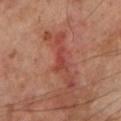The patient is a male approximately 70 years of age. The recorded lesion diameter is about 3 mm. This is a cross-polarized tile. The lesion is on the left lower leg. Cropped from a total-body skin-imaging series; the visible field is about 15 mm.